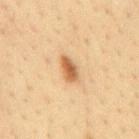This lesion was catalogued during total-body skin photography and was not selected for biopsy.
From the back.
A lesion tile, about 15 mm wide, cut from a 3D total-body photograph.
The subject is a male aged approximately 35.